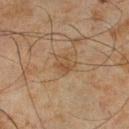Captured during whole-body skin photography for melanoma surveillance; the lesion was not biopsied. Measured at roughly 2.5 mm in maximum diameter. A 15 mm close-up extracted from a 3D total-body photography capture. A male patient in their mid-40s. The lesion is on the right lower leg.